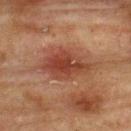No biopsy was performed on this lesion — it was imaged during a full skin examination and was not determined to be concerning. An algorithmic analysis of the crop reported a mean CIELAB color near L≈35 a*≈22 b*≈26, about 9 CIELAB-L* units darker than the surrounding skin, and a lesion-to-skin contrast of about 8 (normalized; higher = more distinct). The software also gave a nevus-likeness score of about 85/100 and lesion-presence confidence of about 100/100. Imaged with cross-polarized lighting. A 15 mm crop from a total-body photograph taken for skin-cancer surveillance. A male patient, aged 73–77. The lesion is located on the back.